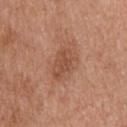* follow-up — catalogued during a skin exam; not biopsied
* tile lighting — white-light illumination
* image — 15 mm crop, total-body photography
* patient — male, aged 58–62
* location — the upper back
* automated metrics — roughly 8 lightness units darker than nearby skin and a lesion-to-skin contrast of about 6 (normalized; higher = more distinct)
* lesion size — about 4 mm